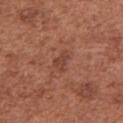Clinical impression: Captured during whole-body skin photography for melanoma surveillance; the lesion was not biopsied.